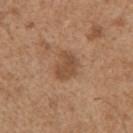workup: catalogued during a skin exam; not biopsied
patient: male, in their mid-60s
image: total-body-photography crop, ~15 mm field of view
anatomic site: the right upper arm